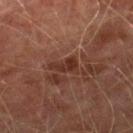<record>
<biopsy_status>not biopsied; imaged during a skin examination</biopsy_status>
<image>
  <source>total-body photography crop</source>
  <field_of_view_mm>15</field_of_view_mm>
</image>
<lesion_size>
  <long_diameter_mm_approx>3.5</long_diameter_mm_approx>
</lesion_size>
<lighting>cross-polarized</lighting>
<site>leg</site>
<patient>
  <sex>male</sex>
  <age_approx>75</age_approx>
</patient>
</record>